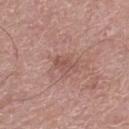body site = the right lower leg; lesion size = ≈3 mm; image = total-body-photography crop, ~15 mm field of view; lighting = white-light illumination; patient = male, in their mid-70s.